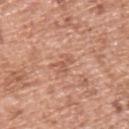Q: Was this lesion biopsied?
A: total-body-photography surveillance lesion; no biopsy
Q: What is the imaging modality?
A: 15 mm crop, total-body photography
Q: What are the patient's age and sex?
A: male, aged 53 to 57
Q: What is the anatomic site?
A: the upper back